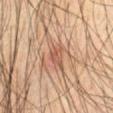biopsy status: no biopsy performed (imaged during a skin exam)
lesion diameter: ≈3 mm
site: the abdomen
image source: ~15 mm tile from a whole-body skin photo
lighting: cross-polarized
patient: male, roughly 65 years of age